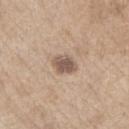tile lighting=white-light; anatomic site=the left upper arm; size=~3 mm (longest diameter); subject=male, roughly 70 years of age; imaging modality=~15 mm tile from a whole-body skin photo.